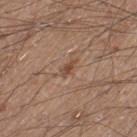Assessment:
Imaged during a routine full-body skin examination; the lesion was not biopsied and no histopathology is available.
Context:
This image is a 15 mm lesion crop taken from a total-body photograph. This is a white-light tile. A male patient, in their 20s. Measured at roughly 2.5 mm in maximum diameter. From the right lower leg.